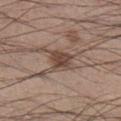No biopsy was performed on this lesion — it was imaged during a full skin examination and was not determined to be concerning. The recorded lesion diameter is about 3 mm. A male patient in their mid- to late 50s. On the right lower leg. Imaged with white-light lighting. Cropped from a total-body skin-imaging series; the visible field is about 15 mm.